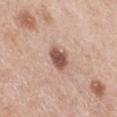{"biopsy_status": "not biopsied; imaged during a skin examination", "site": "right thigh", "image": {"source": "total-body photography crop", "field_of_view_mm": 15}, "automated_metrics": {"cielab_L": 53, "cielab_a": 22, "cielab_b": 26, "vs_skin_contrast_norm": 10.5}, "lighting": "white-light", "patient": {"sex": "female", "age_approx": 65}}